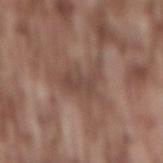Findings:
• workup: total-body-photography surveillance lesion; no biopsy
• patient: male, approximately 75 years of age
• image: ~15 mm tile from a whole-body skin photo
• location: the mid back
• lesion size: about 5 mm
• illumination: white-light